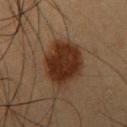Background:
The lesion is located on the left upper arm. The recorded lesion diameter is about 6 mm. This image is a 15 mm lesion crop taken from a total-body photograph. An algorithmic analysis of the crop reported a border-irregularity index near 2/10 and radial color variation of about 1. A male subject, in their mid- to late 50s. This is a cross-polarized tile.